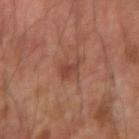Assessment: Captured during whole-body skin photography for melanoma surveillance; the lesion was not biopsied. Clinical summary: A 15 mm close-up extracted from a 3D total-body photography capture. This is a cross-polarized tile. From the right forearm. The subject is a male roughly 60 years of age.